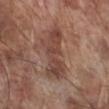<lesion>
<biopsy_status>not biopsied; imaged during a skin examination</biopsy_status>
<image>
  <source>total-body photography crop</source>
  <field_of_view_mm>15</field_of_view_mm>
</image>
<lighting>white-light</lighting>
<patient>
  <sex>male</sex>
  <age_approx>70</age_approx>
</patient>
<site>right lower leg</site>
<lesion_size>
  <long_diameter_mm_approx>6.5</long_diameter_mm_approx>
</lesion_size>
</lesion>